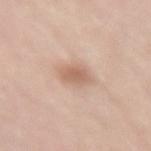{
  "site": "right thigh",
  "lighting": "white-light",
  "automated_metrics": {
    "border_irregularity_0_10": 1.5
  },
  "image": {
    "source": "total-body photography crop",
    "field_of_view_mm": 15
  },
  "lesion_size": {
    "long_diameter_mm_approx": 2.5
  },
  "patient": {
    "sex": "male",
    "age_approx": 65
  }
}